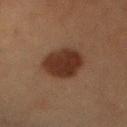notes: imaged on a skin check; not biopsied
image source: ~15 mm tile from a whole-body skin photo
patient: female, aged around 60
automated metrics: a lesion color around L≈26 a*≈17 b*≈23 in CIELAB, about 10 CIELAB-L* units darker than the surrounding skin, and a lesion-to-skin contrast of about 11 (normalized; higher = more distinct); a classifier nevus-likeness of about 95/100 and a lesion-detection confidence of about 100/100
tile lighting: cross-polarized illumination
body site: the mid back